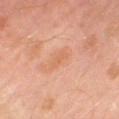biopsy_status: not biopsied; imaged during a skin examination
lesion_size:
  long_diameter_mm_approx: 2.5
image:
  source: total-body photography crop
  field_of_view_mm: 15
site: leg
lighting: cross-polarized
patient:
  sex: male
  age_approx: 55
automated_metrics:
  cielab_L: 62
  cielab_a: 24
  cielab_b: 35
  vs_skin_darker_L: 6.0
  vs_skin_contrast_norm: 4.5